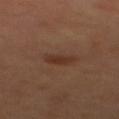Q: Was a biopsy performed?
A: catalogued during a skin exam; not biopsied
Q: Where on the body is the lesion?
A: the upper back
Q: How was this image acquired?
A: total-body-photography crop, ~15 mm field of view
Q: What did automated image analysis measure?
A: a border-irregularity index near 2.5/10, a color-variation rating of about 2/10, and radial color variation of about 0.5
Q: Illumination type?
A: cross-polarized illumination
Q: Patient demographics?
A: female
Q: Lesion size?
A: ≈2.5 mm